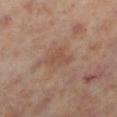* image — ~15 mm tile from a whole-body skin photo
* patient — female, about 55 years old
* body site — the left lower leg
* illumination — cross-polarized